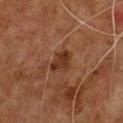follow-up = total-body-photography surveillance lesion; no biopsy | image = total-body-photography crop, ~15 mm field of view | site = the chest | subject = male, approximately 60 years of age.